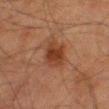Imaged during a routine full-body skin examination; the lesion was not biopsied and no histopathology is available. The subject is a male approximately 80 years of age. The recorded lesion diameter is about 3.5 mm. A 15 mm crop from a total-body photograph taken for skin-cancer surveillance. Imaged with cross-polarized lighting. The total-body-photography lesion software estimated a border-irregularity rating of about 2/10 and peripheral color asymmetry of about 1. On the right thigh.